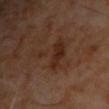Findings:
• notes · no biopsy performed (imaged during a skin exam)
• image source · ~15 mm crop, total-body skin-cancer survey
• subject · male, aged approximately 60
• location · the chest